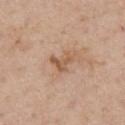Q: Was a biopsy performed?
A: imaged on a skin check; not biopsied
Q: How was this image acquired?
A: 15 mm crop, total-body photography
Q: Lesion size?
A: about 3 mm
Q: What are the patient's age and sex?
A: male, approximately 55 years of age
Q: Where on the body is the lesion?
A: the chest
Q: How was the tile lit?
A: white-light illumination